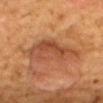diameter=≈7.5 mm; anatomic site=the mid back; image source=15 mm crop, total-body photography; patient=female, about 50 years old; illumination=cross-polarized illumination.